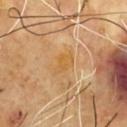| feature | finding |
|---|---|
| notes | total-body-photography surveillance lesion; no biopsy |
| subject | male, in their mid-60s |
| lesion size | ≈3 mm |
| automated lesion analysis | an outline eccentricity of about 0.8 (0 = round, 1 = elongated) and a shape-asymmetry score of about 0.35 (0 = symmetric); a mean CIELAB color near L≈61 a*≈20 b*≈46 and a lesion–skin lightness drop of about 5; border irregularity of about 3.5 on a 0–10 scale, a color-variation rating of about 3.5/10, and a peripheral color-asymmetry measure near 1.5 |
| imaging modality | ~15 mm crop, total-body skin-cancer survey |
| location | the chest |